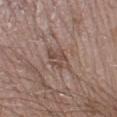A male patient approximately 20 years of age.
Located on the left lower leg.
This is a white-light tile.
Cropped from a total-body skin-imaging series; the visible field is about 15 mm.
The lesion's longest dimension is about 3 mm.